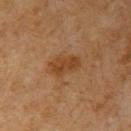Assessment: No biopsy was performed on this lesion — it was imaged during a full skin examination and was not determined to be concerning. Background: A male patient, aged 58–62. A region of skin cropped from a whole-body photographic capture, roughly 15 mm wide. The tile uses cross-polarized illumination. Automated tile analysis of the lesion measured a shape eccentricity near 0.8 and a symmetry-axis asymmetry near 0.2. The software also gave border irregularity of about 2 on a 0–10 scale, a color-variation rating of about 2.5/10, and radial color variation of about 1. The recorded lesion diameter is about 4 mm. From the left upper arm.